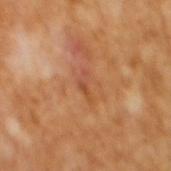Q: Is there a histopathology result?
A: imaged on a skin check; not biopsied
Q: How was this image acquired?
A: ~15 mm crop, total-body skin-cancer survey
Q: Illumination type?
A: cross-polarized illumination
Q: Patient demographics?
A: male, aged 63 to 67